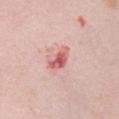The lesion was tiled from a total-body skin photograph and was not biopsied. The lesion is on the chest. Cropped from a total-body skin-imaging series; the visible field is about 15 mm. Automated image analysis of the tile measured a lesion area of about 5.5 mm². The software also gave a lesion color around L≈62 a*≈30 b*≈24 in CIELAB and roughly 13 lightness units darker than nearby skin. A female subject aged approximately 50.